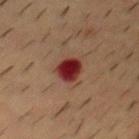{"biopsy_status": "not biopsied; imaged during a skin examination", "patient": {"sex": "male", "age_approx": 55}, "site": "mid back", "automated_metrics": {"eccentricity": 0.7, "shape_asymmetry": 0.2, "vs_skin_darker_L": 15.0, "vs_skin_contrast_norm": 14.5, "border_irregularity_0_10": 1.5, "color_variation_0_10": 3.0, "peripheral_color_asymmetry": 1.0}, "lesion_size": {"long_diameter_mm_approx": 3.5}, "lighting": "cross-polarized", "image": {"source": "total-body photography crop", "field_of_view_mm": 15}}